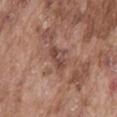The lesion was tiled from a total-body skin photograph and was not biopsied. Captured under white-light illumination. Located on the front of the torso. A close-up tile cropped from a whole-body skin photograph, about 15 mm across. The subject is a male aged approximately 75. Measured at roughly 3.5 mm in maximum diameter.